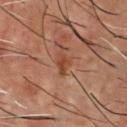The lesion was photographed on a routine skin check and not biopsied; there is no pathology result.
Measured at roughly 3 mm in maximum diameter.
An algorithmic analysis of the crop reported an area of roughly 3.5 mm² and an eccentricity of roughly 0.8. It also reported a mean CIELAB color near L≈43 a*≈24 b*≈32, a lesion–skin lightness drop of about 9, and a normalized lesion–skin contrast near 7.5. It also reported a nevus-likeness score of about 0/100 and lesion-presence confidence of about 100/100.
From the chest.
A roughly 15 mm field-of-view crop from a total-body skin photograph.
A male subject roughly 55 years of age.